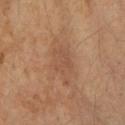{
  "biopsy_status": "not biopsied; imaged during a skin examination",
  "site": "left forearm",
  "image": {
    "source": "total-body photography crop",
    "field_of_view_mm": 15
  },
  "patient": {
    "sex": "female",
    "age_approx": 60
  },
  "lesion_size": {
    "long_diameter_mm_approx": 6.0
  },
  "lighting": "cross-polarized"
}